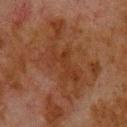Assessment: Recorded during total-body skin imaging; not selected for excision or biopsy. Background: Measured at roughly 9 mm in maximum diameter. The lesion is located on the back. This image is a 15 mm lesion crop taken from a total-body photograph. The total-body-photography lesion software estimated a footprint of about 26 mm², an outline eccentricity of about 0.9 (0 = round, 1 = elongated), and a shape-asymmetry score of about 0.55 (0 = symmetric). The patient is a male aged 78–82.